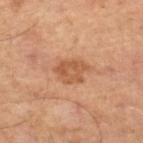Imaged during a routine full-body skin examination; the lesion was not biopsied and no histopathology is available.
Cropped from a total-body skin-imaging series; the visible field is about 15 mm.
The lesion's longest dimension is about 4 mm.
The patient is a male aged approximately 50.
This is a cross-polarized tile.
Located on the left leg.